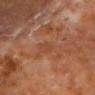The recorded lesion diameter is about 1.5 mm. The tile uses cross-polarized illumination. The patient is a male about 65 years old. From the head or neck. This image is a 15 mm lesion crop taken from a total-body photograph.